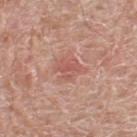| key | value |
|---|---|
| location | the right lower leg |
| image-analysis metrics | an eccentricity of roughly 0.5 and a shape-asymmetry score of about 0.35 (0 = symmetric); about 8 CIELAB-L* units darker than the surrounding skin and a normalized lesion–skin contrast near 5.5 |
| patient | male, about 80 years old |
| tile lighting | white-light illumination |
| imaging modality | ~15 mm crop, total-body skin-cancer survey |
| lesion diameter | ~2.5 mm (longest diameter) |